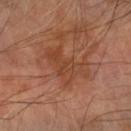The lesion was tiled from a total-body skin photograph and was not biopsied.
Automated tile analysis of the lesion measured a lesion area of about 13 mm² and a symmetry-axis asymmetry near 0.5. And it measured an automated nevus-likeness rating near 0 out of 100 and a detector confidence of about 100 out of 100 that the crop contains a lesion.
Cropped from a total-body skin-imaging series; the visible field is about 15 mm.
On the left forearm.
Imaged with cross-polarized lighting.
The subject is a male about 70 years old.